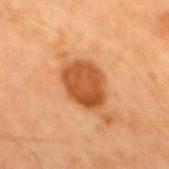notes = total-body-photography surveillance lesion; no biopsy
diameter = about 5 mm
site = the mid back
patient = male, approximately 65 years of age
imaging modality = ~15 mm tile from a whole-body skin photo
tile lighting = cross-polarized